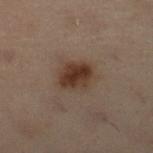Recorded during total-body skin imaging; not selected for excision or biopsy. The subject is a female aged approximately 55. The lesion is on the left leg. Cropped from a whole-body photographic skin survey; the tile spans about 15 mm. Imaged with cross-polarized lighting. The recorded lesion diameter is about 3.5 mm.